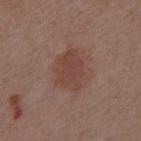The lesion was tiled from a total-body skin photograph and was not biopsied. Cropped from a whole-body photographic skin survey; the tile spans about 15 mm. The subject is a male about 55 years old. The lesion is located on the chest.